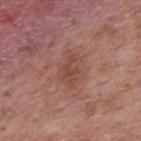The patient is a female aged around 60.
The lesion is located on the upper back.
Cropped from a total-body skin-imaging series; the visible field is about 15 mm.